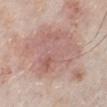Case summary:
• follow-up · no biopsy performed (imaged during a skin exam)
• image source · total-body-photography crop, ~15 mm field of view
• lighting · white-light
• body site · the left lower leg
• lesion diameter · about 10.5 mm
• subject · male, aged 78 to 82
• image-analysis metrics · a classifier nevus-likeness of about 0/100 and a lesion-detection confidence of about 90/100